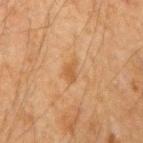No biopsy was performed on this lesion — it was imaged during a full skin examination and was not determined to be concerning.
This is a cross-polarized tile.
A male subject in their 50s.
A 15 mm close-up tile from a total-body photography series done for melanoma screening.
The lesion is on the right upper arm.